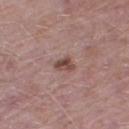notes: imaged on a skin check; not biopsied | patient: male, aged 48–52 | anatomic site: the right thigh | lesion size: ≈2.5 mm | automated lesion analysis: a mean CIELAB color near L≈47 a*≈20 b*≈22, roughly 11 lightness units darker than nearby skin, and a normalized border contrast of about 8.5; lesion-presence confidence of about 100/100 | imaging modality: ~15 mm crop, total-body skin-cancer survey.